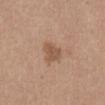| feature | finding |
|---|---|
| follow-up | imaged on a skin check; not biopsied |
| patient | male, in their mid- to late 20s |
| automated lesion analysis | roughly 9 lightness units darker than nearby skin and a lesion-to-skin contrast of about 6.5 (normalized; higher = more distinct); border irregularity of about 3 on a 0–10 scale and a peripheral color-asymmetry measure near 0.5; a classifier nevus-likeness of about 10/100 and a lesion-detection confidence of about 100/100 |
| illumination | white-light |
| imaging modality | ~15 mm crop, total-body skin-cancer survey |
| diameter | ≈2.5 mm |
| body site | the abdomen |